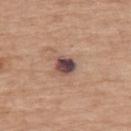This lesion was catalogued during total-body skin photography and was not selected for biopsy. A 15 mm close-up extracted from a 3D total-body photography capture. From the back. The subject is a male roughly 75 years of age.